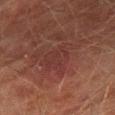Q: What are the patient's age and sex?
A: male, aged 73 to 77
Q: What is the imaging modality?
A: 15 mm crop, total-body photography
Q: Lesion location?
A: the right thigh